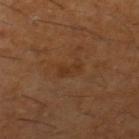Clinical impression: The lesion was tiled from a total-body skin photograph and was not biopsied. Image and clinical context: The recorded lesion diameter is about 3 mm. The lesion-visualizer software estimated a footprint of about 4 mm². The analysis additionally found a color-variation rating of about 1.5/10. And it measured a classifier nevus-likeness of about 0/100 and lesion-presence confidence of about 100/100. A close-up tile cropped from a whole-body skin photograph, about 15 mm across. A male subject aged 58 to 62. Located on the left thigh.